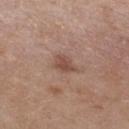follow-up: total-body-photography surveillance lesion; no biopsy | patient: female, approximately 40 years of age | lighting: white-light illumination | image source: ~15 mm tile from a whole-body skin photo | anatomic site: the chest.